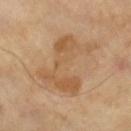follow-up=catalogued during a skin exam; not biopsied
image=~15 mm crop, total-body skin-cancer survey
patient=male, aged 63–67
size=about 7 mm